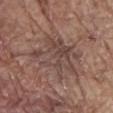| key | value |
|---|---|
| workup | imaged on a skin check; not biopsied |
| image source | 15 mm crop, total-body photography |
| subject | male, aged approximately 80 |
| location | the front of the torso |